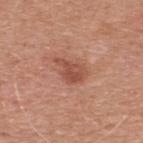biopsy status = total-body-photography surveillance lesion; no biopsy | subject = male, approximately 50 years of age | body site = the upper back | imaging modality = ~15 mm tile from a whole-body skin photo.